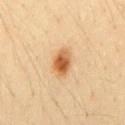Assessment: The lesion was photographed on a routine skin check and not biopsied; there is no pathology result. Clinical summary: A lesion tile, about 15 mm wide, cut from a 3D total-body photograph. The lesion-visualizer software estimated an area of roughly 6.5 mm², an outline eccentricity of about 0.75 (0 = round, 1 = elongated), and two-axis asymmetry of about 0.15. The software also gave a lesion color around L≈60 a*≈23 b*≈41 in CIELAB and a normalized border contrast of about 9.5. A male patient aged around 45. The lesion is located on the mid back. Captured under cross-polarized illumination. The lesion's longest dimension is about 3.5 mm.